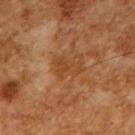patient = male, approximately 65 years of age; lighting = cross-polarized; image source = 15 mm crop, total-body photography; lesion size = ~3 mm (longest diameter).